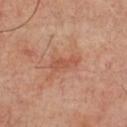Captured during whole-body skin photography for melanoma surveillance; the lesion was not biopsied. From the chest. Longest diameter approximately 3.5 mm. A subject in their mid- to late 50s. This image is a 15 mm lesion crop taken from a total-body photograph.